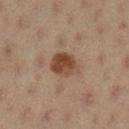The lesion was tiled from a total-body skin photograph and was not biopsied. A female subject roughly 20 years of age. A 15 mm close-up extracted from a 3D total-body photography capture. The lesion-visualizer software estimated an area of roughly 8 mm², an outline eccentricity of about 0.6 (0 = round, 1 = elongated), and two-axis asymmetry of about 0.15. And it measured a lesion color around L≈38 a*≈18 b*≈26 in CIELAB and a normalized border contrast of about 9.5. The software also gave a nevus-likeness score of about 90/100 and lesion-presence confidence of about 100/100. The lesion is located on the left lower leg. Approximately 3.5 mm at its widest. Captured under cross-polarized illumination.